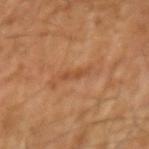Findings:
- illumination — cross-polarized
- subject — male, approximately 65 years of age
- lesion diameter — ≈3 mm
- acquisition — ~15 mm crop, total-body skin-cancer survey